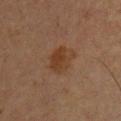Recorded during total-body skin imaging; not selected for excision or biopsy.
A region of skin cropped from a whole-body photographic capture, roughly 15 mm wide.
The lesion is located on the chest.
Automated tile analysis of the lesion measured a mean CIELAB color near L≈31 a*≈16 b*≈26, a lesion–skin lightness drop of about 6, and a normalized border contrast of about 7. The software also gave an automated nevus-likeness rating near 55 out of 100 and a detector confidence of about 100 out of 100 that the crop contains a lesion.
The patient is a male aged approximately 60.